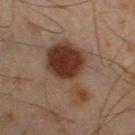Part of a total-body skin-imaging series; this lesion was reviewed on a skin check and was not flagged for biopsy. A 15 mm close-up extracted from a 3D total-body photography capture. Imaged with cross-polarized lighting. About 8 mm across. The lesion-visualizer software estimated an outline eccentricity of about 0.9 (0 = round, 1 = elongated). It also reported a lesion color around L≈29 a*≈16 b*≈22 in CIELAB and about 11 CIELAB-L* units darker than the surrounding skin. It also reported a nevus-likeness score of about 100/100 and a detector confidence of about 100 out of 100 that the crop contains a lesion. A male subject, aged around 45. The lesion is located on the right thigh.